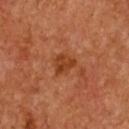Q: Was a biopsy performed?
A: total-body-photography surveillance lesion; no biopsy
Q: What is the lesion's diameter?
A: ~3 mm (longest diameter)
Q: What is the imaging modality?
A: 15 mm crop, total-body photography
Q: Lesion location?
A: the chest
Q: Automated lesion metrics?
A: a footprint of about 5 mm², an eccentricity of roughly 0.55, and a symmetry-axis asymmetry near 0.35; a normalized lesion–skin contrast near 7.5; a classifier nevus-likeness of about 10/100 and a detector confidence of about 100 out of 100 that the crop contains a lesion
Q: How was the tile lit?
A: cross-polarized illumination
Q: Patient demographics?
A: female, about 65 years old